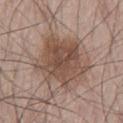Recorded during total-body skin imaging; not selected for excision or biopsy. A male patient in their mid-50s. The lesion is located on the left thigh. A 15 mm crop from a total-body photograph taken for skin-cancer surveillance.